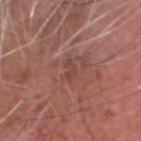Imaged during a routine full-body skin examination; the lesion was not biopsied and no histopathology is available. The lesion's longest dimension is about 2.5 mm. A 15 mm close-up extracted from a 3D total-body photography capture. A male subject in their mid-70s. The total-body-photography lesion software estimated a footprint of about 2 mm², an outline eccentricity of about 0.9 (0 = round, 1 = elongated), and two-axis asymmetry of about 0.5. Imaged with white-light lighting. The lesion is located on the head or neck.